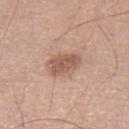Q: Was this lesion biopsied?
A: imaged on a skin check; not biopsied
Q: What did automated image analysis measure?
A: an area of roughly 8.5 mm², an outline eccentricity of about 0.8 (0 = round, 1 = elongated), and a shape-asymmetry score of about 0.2 (0 = symmetric); an average lesion color of about L≈56 a*≈20 b*≈28 (CIELAB), about 11 CIELAB-L* units darker than the surrounding skin, and a lesion-to-skin contrast of about 7.5 (normalized; higher = more distinct); border irregularity of about 2 on a 0–10 scale and internal color variation of about 2.5 on a 0–10 scale; a nevus-likeness score of about 45/100 and lesion-presence confidence of about 100/100
Q: What are the patient's age and sex?
A: male, about 55 years old
Q: What is the imaging modality?
A: total-body-photography crop, ~15 mm field of view
Q: What is the lesion's diameter?
A: ≈4 mm
Q: What is the anatomic site?
A: the left lower leg